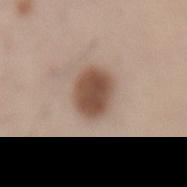Part of a total-body skin-imaging series; this lesion was reviewed on a skin check and was not flagged for biopsy. Automated image analysis of the tile measured an average lesion color of about L≈50 a*≈17 b*≈27 (CIELAB), about 15 CIELAB-L* units darker than the surrounding skin, and a normalized border contrast of about 10.5. And it measured radial color variation of about 1. The lesion's longest dimension is about 4.5 mm. The subject is a male about 55 years old. Captured under white-light illumination. Located on the back. A close-up tile cropped from a whole-body skin photograph, about 15 mm across.